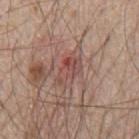Assessment: No biopsy was performed on this lesion — it was imaged during a full skin examination and was not determined to be concerning. Background: About 3.5 mm across. Captured under white-light illumination. A 15 mm close-up extracted from a 3D total-body photography capture. Automated tile analysis of the lesion measured a lesion area of about 6 mm² and a shape eccentricity near 0.8. And it measured a lesion–skin lightness drop of about 8 and a normalized border contrast of about 6. Located on the mid back. The subject is a male roughly 65 years of age.